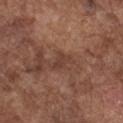No biopsy was performed on this lesion — it was imaged during a full skin examination and was not determined to be concerning. The tile uses white-light illumination. The lesion is on the chest. A male patient about 75 years old. The lesion's longest dimension is about 3 mm. A 15 mm close-up tile from a total-body photography series done for melanoma screening. The lesion-visualizer software estimated border irregularity of about 4.5 on a 0–10 scale and a color-variation rating of about 1.5/10.